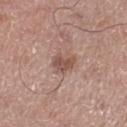biopsy status: catalogued during a skin exam; not biopsied | subject: male, aged 58–62 | image-analysis metrics: an area of roughly 5.5 mm², a shape eccentricity near 0.65, and a shape-asymmetry score of about 0.25 (0 = symmetric); a mean CIELAB color near L≈52 a*≈20 b*≈25 and a lesion-to-skin contrast of about 7 (normalized; higher = more distinct); a classifier nevus-likeness of about 30/100 and lesion-presence confidence of about 100/100 | imaging modality: ~15 mm tile from a whole-body skin photo | anatomic site: the right lower leg | lesion diameter: ~3 mm (longest diameter).